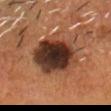This lesion was catalogued during total-body skin photography and was not selected for biopsy. About 6.5 mm across. The total-body-photography lesion software estimated an area of roughly 25 mm², an outline eccentricity of about 0.65 (0 = round, 1 = elongated), and two-axis asymmetry of about 0.15. The software also gave an average lesion color of about L≈31 a*≈18 b*≈25 (CIELAB), roughly 18 lightness units darker than nearby skin, and a normalized border contrast of about 15.5. It also reported border irregularity of about 2 on a 0–10 scale, a within-lesion color-variation index near 8/10, and peripheral color asymmetry of about 2. Cropped from a whole-body photographic skin survey; the tile spans about 15 mm. Captured under cross-polarized illumination. From the head or neck. A male subject roughly 55 years of age.